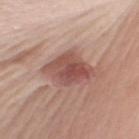Findings:
* biopsy status · no biopsy performed (imaged during a skin exam)
* subject · female, aged 63 to 67
* lighting · white-light illumination
* lesion diameter · ≈5 mm
* site · the back
* image · ~15 mm tile from a whole-body skin photo